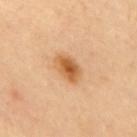Context:
The recorded lesion diameter is about 4 mm. A 15 mm close-up extracted from a 3D total-body photography capture. The lesion is on the back. The lesion-visualizer software estimated a lesion color around L≈61 a*≈24 b*≈43 in CIELAB. The software also gave border irregularity of about 2 on a 0–10 scale, internal color variation of about 4.5 on a 0–10 scale, and a peripheral color-asymmetry measure near 1.